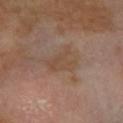The lesion was tiled from a total-body skin photograph and was not biopsied. From the right forearm. A male patient aged 53–57. Longest diameter approximately 3.5 mm. Cropped from a total-body skin-imaging series; the visible field is about 15 mm. Imaged with cross-polarized lighting.